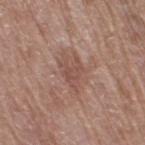{"biopsy_status": "not biopsied; imaged during a skin examination", "site": "right thigh", "patient": {"sex": "female", "age_approx": 80}, "automated_metrics": {"area_mm2_approx": 4.5, "eccentricity": 0.7, "shape_asymmetry": 0.5, "nevus_likeness_0_100": 0, "lesion_detection_confidence_0_100": 100}, "lighting": "white-light", "image": {"source": "total-body photography crop", "field_of_view_mm": 15}}